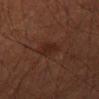Q: Is there a histopathology result?
A: no biopsy performed (imaged during a skin exam)
Q: Who is the patient?
A: male, aged 63–67
Q: What is the imaging modality?
A: 15 mm crop, total-body photography
Q: Where on the body is the lesion?
A: the right thigh